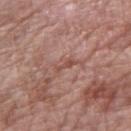Impression:
The lesion was tiled from a total-body skin photograph and was not biopsied.
Acquisition and patient details:
On the arm. A 15 mm close-up tile from a total-body photography series done for melanoma screening. Imaged with white-light lighting. A female subject aged approximately 60. The total-body-photography lesion software estimated a lesion area of about 2.5 mm², an outline eccentricity of about 0.95 (0 = round, 1 = elongated), and a symmetry-axis asymmetry near 0.4. It also reported a lesion–skin lightness drop of about 8. The software also gave border irregularity of about 4.5 on a 0–10 scale, internal color variation of about 0 on a 0–10 scale, and a peripheral color-asymmetry measure near 0. It also reported a nevus-likeness score of about 0/100 and a lesion-detection confidence of about 70/100.